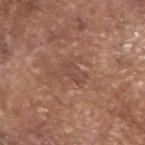The lesion was photographed on a routine skin check and not biopsied; there is no pathology result.
Automated image analysis of the tile measured a footprint of about 2 mm², an eccentricity of roughly 0.9, and a symmetry-axis asymmetry near 0.7. It also reported an average lesion color of about L≈46 a*≈22 b*≈27 (CIELAB) and a normalized border contrast of about 4.5. The analysis additionally found border irregularity of about 7 on a 0–10 scale and a color-variation rating of about 0/10. The software also gave an automated nevus-likeness rating near 0 out of 100 and a lesion-detection confidence of about 100/100.
About 2.5 mm across.
From the head or neck.
Imaged with white-light lighting.
A male subject aged around 80.
A lesion tile, about 15 mm wide, cut from a 3D total-body photograph.